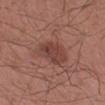Q: Was a biopsy performed?
A: no biopsy performed (imaged during a skin exam)
Q: What lighting was used for the tile?
A: white-light
Q: How was this image acquired?
A: total-body-photography crop, ~15 mm field of view
Q: Where on the body is the lesion?
A: the arm
Q: Patient demographics?
A: male, in their mid-30s
Q: Automated lesion metrics?
A: a lesion-to-skin contrast of about 7 (normalized; higher = more distinct)
Q: How large is the lesion?
A: ~4.5 mm (longest diameter)